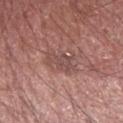Captured during whole-body skin photography for melanoma surveillance; the lesion was not biopsied. Automated tile analysis of the lesion measured a footprint of about 5.5 mm², an eccentricity of roughly 0.8, and a shape-asymmetry score of about 0.4 (0 = symmetric). And it measured an automated nevus-likeness rating near 0 out of 100 and a detector confidence of about 100 out of 100 that the crop contains a lesion. The recorded lesion diameter is about 3.5 mm. A lesion tile, about 15 mm wide, cut from a 3D total-body photograph. The subject is a male aged 73–77. The lesion is located on the arm.